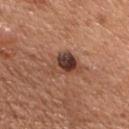The lesion was photographed on a routine skin check and not biopsied; there is no pathology result. This is a white-light tile. The patient is a male aged approximately 55. Located on the chest. Cropped from a total-body skin-imaging series; the visible field is about 15 mm.